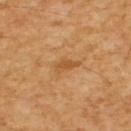notes=total-body-photography surveillance lesion; no biopsy | automated metrics=border irregularity of about 3.5 on a 0–10 scale; an automated nevus-likeness rating near 5 out of 100 and a detector confidence of about 100 out of 100 that the crop contains a lesion | imaging modality=15 mm crop, total-body photography | patient=male, in their 60s | anatomic site=the upper back | diameter=≈3 mm | tile lighting=cross-polarized.